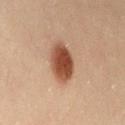Part of a total-body skin-imaging series; this lesion was reviewed on a skin check and was not flagged for biopsy. The patient is a female in their 30s. An algorithmic analysis of the crop reported a shape eccentricity near 0.7 and a shape-asymmetry score of about 0.1 (0 = symmetric). And it measured a classifier nevus-likeness of about 100/100 and a lesion-detection confidence of about 100/100. A roughly 15 mm field-of-view crop from a total-body skin photograph. The tile uses cross-polarized illumination. On the abdomen.